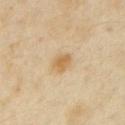Captured during whole-body skin photography for melanoma surveillance; the lesion was not biopsied. Cropped from a total-body skin-imaging series; the visible field is about 15 mm. A male patient, aged around 50. The lesion is located on the left upper arm. The lesion's longest dimension is about 2.5 mm. The tile uses cross-polarized illumination. The lesion-visualizer software estimated a footprint of about 4.5 mm² and an eccentricity of roughly 0.65. It also reported a lesion color around L≈63 a*≈15 b*≈40 in CIELAB, a lesion–skin lightness drop of about 9, and a normalized border contrast of about 7.5. It also reported a detector confidence of about 100 out of 100 that the crop contains a lesion.